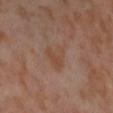Part of a total-body skin-imaging series; this lesion was reviewed on a skin check and was not flagged for biopsy.
A close-up tile cropped from a whole-body skin photograph, about 15 mm across.
The subject is a female in their mid-50s.
The recorded lesion diameter is about 4 mm.
The lesion is located on the left thigh.
The tile uses cross-polarized illumination.
Automated image analysis of the tile measured internal color variation of about 2 on a 0–10 scale and peripheral color asymmetry of about 0.5.